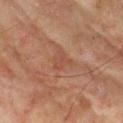Clinical impression: Part of a total-body skin-imaging series; this lesion was reviewed on a skin check and was not flagged for biopsy. Acquisition and patient details: From the upper back. Cropped from a total-body skin-imaging series; the visible field is about 15 mm. A male patient, approximately 75 years of age.